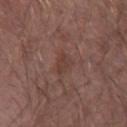| key | value |
|---|---|
| follow-up | imaged on a skin check; not biopsied |
| patient | male, approximately 65 years of age |
| TBP lesion metrics | a footprint of about 3.5 mm², an eccentricity of roughly 0.85, and a shape-asymmetry score of about 0.25 (0 = symmetric); a border-irregularity index near 2.5/10, a color-variation rating of about 2.5/10, and a peripheral color-asymmetry measure near 1; a classifier nevus-likeness of about 0/100 and lesion-presence confidence of about 100/100 |
| lighting | white-light illumination |
| acquisition | total-body-photography crop, ~15 mm field of view |
| anatomic site | the left forearm |
| lesion size | ≈2.5 mm |